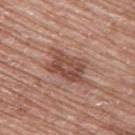follow-up: imaged on a skin check; not biopsied
acquisition: ~15 mm crop, total-body skin-cancer survey
lighting: white-light
size: about 5.5 mm
subject: female, aged approximately 60
body site: the upper back